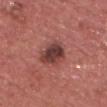Impression:
This lesion was catalogued during total-body skin photography and was not selected for biopsy.
Context:
This is a white-light tile. A male subject about 70 years old. The lesion is on the head or neck. A region of skin cropped from a whole-body photographic capture, roughly 15 mm wide. Automated tile analysis of the lesion measured a footprint of about 8.5 mm². The analysis additionally found a mean CIELAB color near L≈40 a*≈25 b*≈22, a lesion–skin lightness drop of about 14, and a lesion-to-skin contrast of about 10.5 (normalized; higher = more distinct). It also reported border irregularity of about 2.5 on a 0–10 scale, internal color variation of about 5 on a 0–10 scale, and radial color variation of about 1.5. The analysis additionally found an automated nevus-likeness rating near 75 out of 100 and a lesion-detection confidence of about 100/100. Approximately 4 mm at its widest.